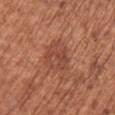The lesion was tiled from a total-body skin photograph and was not biopsied. Cropped from a whole-body photographic skin survey; the tile spans about 15 mm. The lesion is located on the left upper arm. This is a white-light tile. The total-body-photography lesion software estimated border irregularity of about 2.5 on a 0–10 scale, a within-lesion color-variation index near 3.5/10, and peripheral color asymmetry of about 1.5. And it measured an automated nevus-likeness rating near 0 out of 100 and a lesion-detection confidence of about 100/100. A female subject, roughly 75 years of age.